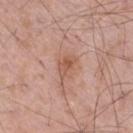From the right thigh. A 15 mm close-up extracted from a 3D total-body photography capture. The patient is a male about 55 years old.